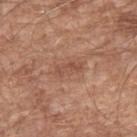Q: Was a biopsy performed?
A: imaged on a skin check; not biopsied
Q: What is the anatomic site?
A: the arm
Q: How was the tile lit?
A: white-light illumination
Q: What is the imaging modality?
A: ~15 mm crop, total-body skin-cancer survey
Q: Who is the patient?
A: male, in their mid- to late 60s
Q: What did automated image analysis measure?
A: border irregularity of about 3 on a 0–10 scale, internal color variation of about 2.5 on a 0–10 scale, and radial color variation of about 1; a lesion-detection confidence of about 95/100
Q: What is the lesion's diameter?
A: ≈4 mm